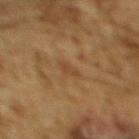follow-up: catalogued during a skin exam; not biopsied | subject: male, aged 83 or older | diameter: ≈3 mm | image: total-body-photography crop, ~15 mm field of view | tile lighting: cross-polarized | site: the back | automated lesion analysis: a lesion color around L≈38 a*≈16 b*≈30 in CIELAB, about 5 CIELAB-L* units darker than the surrounding skin, and a normalized lesion–skin contrast near 5; border irregularity of about 3 on a 0–10 scale, internal color variation of about 1 on a 0–10 scale, and peripheral color asymmetry of about 0.